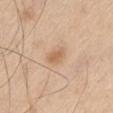biopsy status: no biopsy performed (imaged during a skin exam); lesion size: ≈2.5 mm; image source: ~15 mm crop, total-body skin-cancer survey; lighting: white-light illumination; body site: the left thigh; subject: male, aged 58–62.